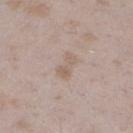This lesion was catalogued during total-body skin photography and was not selected for biopsy. The recorded lesion diameter is about 3 mm. The tile uses white-light illumination. A lesion tile, about 15 mm wide, cut from a 3D total-body photograph. The total-body-photography lesion software estimated an outline eccentricity of about 0.85 (0 = round, 1 = elongated) and a symmetry-axis asymmetry near 0.45. The analysis additionally found a mean CIELAB color near L≈60 a*≈14 b*≈25, a lesion–skin lightness drop of about 7, and a lesion-to-skin contrast of about 5.5 (normalized; higher = more distinct). It also reported a border-irregularity index near 5/10 and a color-variation rating of about 2/10. The lesion is on the left lower leg. A female subject about 25 years old.